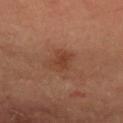Impression: No biopsy was performed on this lesion — it was imaged during a full skin examination and was not determined to be concerning. Context: The tile uses cross-polarized illumination. The lesion is on the right forearm. A male patient, approximately 40 years of age. The total-body-photography lesion software estimated a footprint of about 6 mm², an outline eccentricity of about 0.65 (0 = round, 1 = elongated), and a symmetry-axis asymmetry near 0.25. The software also gave a border-irregularity index near 2.5/10, a color-variation rating of about 2/10, and radial color variation of about 0.5. A close-up tile cropped from a whole-body skin photograph, about 15 mm across.